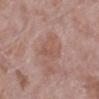This lesion was catalogued during total-body skin photography and was not selected for biopsy.
A female subject aged 68 to 72.
On the left lower leg.
Approximately 3.5 mm at its widest.
Cropped from a whole-body photographic skin survey; the tile spans about 15 mm.